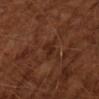Q: Was this lesion biopsied?
A: imaged on a skin check; not biopsied
Q: How large is the lesion?
A: ~3 mm (longest diameter)
Q: Patient demographics?
A: male, aged around 65
Q: How was this image acquired?
A: 15 mm crop, total-body photography
Q: What lighting was used for the tile?
A: cross-polarized illumination